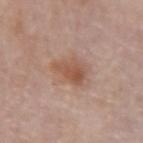Notes:
* imaging modality · 15 mm crop, total-body photography
* location · the right forearm
* subject · female, aged 63 to 67
* diameter · about 4 mm